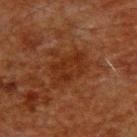Captured during whole-body skin photography for melanoma surveillance; the lesion was not biopsied. A 15 mm close-up tile from a total-body photography series done for melanoma screening. From the upper back. A male subject, roughly 60 years of age.